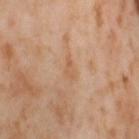This lesion was catalogued during total-body skin photography and was not selected for biopsy. The lesion-visualizer software estimated a lesion color around L≈60 a*≈20 b*≈36 in CIELAB and a normalized border contrast of about 5. And it measured a border-irregularity index near 4/10. The lesion is on the leg. The tile uses cross-polarized illumination. A lesion tile, about 15 mm wide, cut from a 3D total-body photograph. The subject is a female about 55 years old.